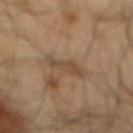workup: catalogued during a skin exam; not biopsied | location: the mid back | size: ~3.5 mm (longest diameter) | acquisition: ~15 mm crop, total-body skin-cancer survey | patient: male, aged around 65.